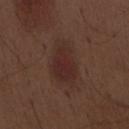Case summary:
* workup — catalogued during a skin exam; not biopsied
* automated metrics — a footprint of about 13 mm², a shape eccentricity near 0.85, and a shape-asymmetry score of about 0.25 (0 = symmetric); a border-irregularity rating of about 3/10, a within-lesion color-variation index near 2.5/10, and radial color variation of about 0.5; a detector confidence of about 100 out of 100 that the crop contains a lesion
* image — 15 mm crop, total-body photography
* location — the abdomen
* tile lighting — white-light illumination
* size — ≈6 mm
* subject — male, aged approximately 70